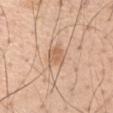Clinical impression:
Captured during whole-body skin photography for melanoma surveillance; the lesion was not biopsied.
Clinical summary:
Imaged with white-light lighting. A 15 mm close-up extracted from a 3D total-body photography capture. The patient is a male aged around 55. The lesion is located on the left upper arm.